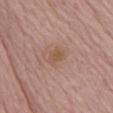notes=catalogued during a skin exam; not biopsied | imaging modality=total-body-photography crop, ~15 mm field of view | lesion diameter=≈2.5 mm | image-analysis metrics=a lesion area of about 3.5 mm² and an eccentricity of roughly 0.8; about 6 CIELAB-L* units darker than the surrounding skin and a normalized lesion–skin contrast near 6; a color-variation rating of about 1/10 and radial color variation of about 0.5; an automated nevus-likeness rating near 0 out of 100 and lesion-presence confidence of about 100/100 | location=the chest | patient=male, aged approximately 85 or older.